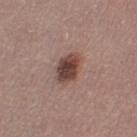<lesion>
  <biopsy_status>not biopsied; imaged during a skin examination</biopsy_status>
  <patient>
    <sex>female</sex>
    <age_approx>35</age_approx>
  </patient>
  <lighting>white-light</lighting>
  <image>
    <source>total-body photography crop</source>
    <field_of_view_mm>15</field_of_view_mm>
  </image>
  <site>left thigh</site>
  <lesion_size>
    <long_diameter_mm_approx>4.0</long_diameter_mm_approx>
  </lesion_size>
</lesion>